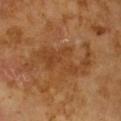Q: Was a biopsy performed?
A: imaged on a skin check; not biopsied
Q: What kind of image is this?
A: ~15 mm crop, total-body skin-cancer survey
Q: How was the tile lit?
A: cross-polarized
Q: What is the anatomic site?
A: the chest
Q: Automated lesion metrics?
A: a nevus-likeness score of about 0/100 and a detector confidence of about 30 out of 100 that the crop contains a lesion
Q: What is the lesion's diameter?
A: ~9 mm (longest diameter)
Q: Who is the patient?
A: female, about 70 years old